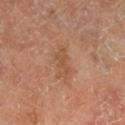This is a cross-polarized tile.
Longest diameter approximately 3.5 mm.
A close-up tile cropped from a whole-body skin photograph, about 15 mm across.
A male patient aged around 65.
Automated image analysis of the tile measured a lesion area of about 5 mm², a shape eccentricity near 0.9, and a symmetry-axis asymmetry near 0.25. It also reported an average lesion color of about L≈52 a*≈22 b*≈33 (CIELAB), about 7 CIELAB-L* units darker than the surrounding skin, and a normalized border contrast of about 5.5. And it measured a nevus-likeness score of about 0/100 and a lesion-detection confidence of about 100/100.
The lesion is on the right lower leg.